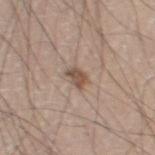Clinical impression:
No biopsy was performed on this lesion — it was imaged during a full skin examination and was not determined to be concerning.
Context:
A male patient, aged around 75. The lesion-visualizer software estimated an area of roughly 3.5 mm², an eccentricity of roughly 0.7, and a shape-asymmetry score of about 0.3 (0 = symmetric). It also reported a mean CIELAB color near L≈51 a*≈16 b*≈26, about 11 CIELAB-L* units darker than the surrounding skin, and a lesion-to-skin contrast of about 8 (normalized; higher = more distinct). The software also gave a border-irregularity index near 3.5/10, internal color variation of about 2 on a 0–10 scale, and radial color variation of about 1. And it measured a nevus-likeness score of about 85/100 and lesion-presence confidence of about 100/100. From the left thigh. A close-up tile cropped from a whole-body skin photograph, about 15 mm across. Imaged with white-light lighting.